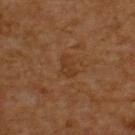Clinical impression: No biopsy was performed on this lesion — it was imaged during a full skin examination and was not determined to be concerning. Acquisition and patient details: Approximately 2.5 mm at its widest. Imaged with cross-polarized lighting. On the upper back. Cropped from a whole-body photographic skin survey; the tile spans about 15 mm. A male patient aged 58 to 62.